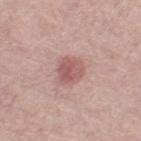No biopsy was performed on this lesion — it was imaged during a full skin examination and was not determined to be concerning. The patient is a female in their mid- to late 60s. This image is a 15 mm lesion crop taken from a total-body photograph. The recorded lesion diameter is about 3 mm. Automated tile analysis of the lesion measured a lesion area of about 8 mm², an outline eccentricity of about 0.3 (0 = round, 1 = elongated), and a shape-asymmetry score of about 0.2 (0 = symmetric). It also reported internal color variation of about 3.5 on a 0–10 scale and a peripheral color-asymmetry measure near 1. It also reported a nevus-likeness score of about 60/100 and a detector confidence of about 100 out of 100 that the crop contains a lesion. Imaged with white-light lighting. On the left thigh.